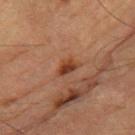Image and clinical context:
Automated tile analysis of the lesion measured roughly 9 lightness units darker than nearby skin. The software also gave a border-irregularity rating of about 3.5/10, a color-variation rating of about 3.5/10, and radial color variation of about 1. On the right thigh. A 15 mm crop from a total-body photograph taken for skin-cancer surveillance. A male subject approximately 85 years of age or older. Imaged with cross-polarized lighting.